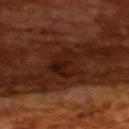Assessment:
The lesion was photographed on a routine skin check and not biopsied; there is no pathology result.
Acquisition and patient details:
A 15 mm crop from a total-body photograph taken for skin-cancer surveillance. A male subject aged 63 to 67. This is a cross-polarized tile. Automated image analysis of the tile measured a lesion area of about 10 mm² and a shape eccentricity near 0.65. It also reported radial color variation of about 1.5. The software also gave a classifier nevus-likeness of about 0/100 and a detector confidence of about 100 out of 100 that the crop contains a lesion. About 4 mm across.